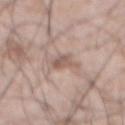workup = catalogued during a skin exam; not biopsied
image source = ~15 mm tile from a whole-body skin photo
lighting = white-light illumination
patient = male, roughly 55 years of age
site = the abdomen